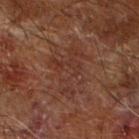Captured during whole-body skin photography for melanoma surveillance; the lesion was not biopsied. A 15 mm close-up tile from a total-body photography series done for melanoma screening. Captured under cross-polarized illumination. On the right forearm. A male subject aged approximately 65. Longest diameter approximately 6 mm.